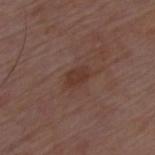Findings:
- notes — total-body-photography surveillance lesion; no biopsy
- site — the chest
- lesion size — about 3 mm
- patient — male, approximately 50 years of age
- automated lesion analysis — a lesion area of about 4 mm², a shape eccentricity near 0.75, and a shape-asymmetry score of about 0.15 (0 = symmetric)
- tile lighting — white-light illumination
- image source — ~15 mm crop, total-body skin-cancer survey